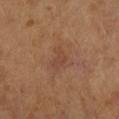{"lighting": "cross-polarized", "image": {"source": "total-body photography crop", "field_of_view_mm": 15}, "patient": {"sex": "female", "age_approx": 60}, "lesion_size": {"long_diameter_mm_approx": 3.0}, "site": "right forearm"}